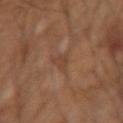Impression: The lesion was photographed on a routine skin check and not biopsied; there is no pathology result. Context: The total-body-photography lesion software estimated a border-irregularity index near 5/10, internal color variation of about 1 on a 0–10 scale, and peripheral color asymmetry of about 0. Imaged with cross-polarized lighting. From the right forearm. Cropped from a total-body skin-imaging series; the visible field is about 15 mm.